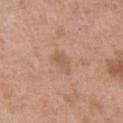Context:
The subject is a female approximately 40 years of age. Imaged with white-light lighting. Cropped from a total-body skin-imaging series; the visible field is about 15 mm. The recorded lesion diameter is about 2.5 mm. The lesion-visualizer software estimated two-axis asymmetry of about 0.2. The software also gave an automated nevus-likeness rating near 0 out of 100 and a lesion-detection confidence of about 100/100. Located on the chest.